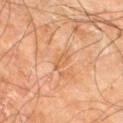| feature | finding |
|---|---|
| workup | total-body-photography surveillance lesion; no biopsy |
| site | the right leg |
| diameter | ≈3 mm |
| subject | male, aged 58 to 62 |
| automated lesion analysis | an area of roughly 2.5 mm² and an eccentricity of roughly 0.95; a lesion color around L≈60 a*≈23 b*≈38 in CIELAB |
| illumination | cross-polarized |
| image | total-body-photography crop, ~15 mm field of view |